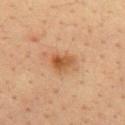Q: Was this lesion biopsied?
A: catalogued during a skin exam; not biopsied
Q: Lesion location?
A: the upper back
Q: What are the patient's age and sex?
A: male, in their mid-30s
Q: How was the tile lit?
A: cross-polarized
Q: Automated lesion metrics?
A: a lesion area of about 6.5 mm² and two-axis asymmetry of about 0.35; a border-irregularity index near 3/10, a color-variation rating of about 5.5/10, and peripheral color asymmetry of about 2; a classifier nevus-likeness of about 75/100
Q: How was this image acquired?
A: 15 mm crop, total-body photography
Q: Lesion size?
A: ≈3 mm